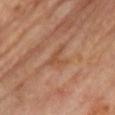{
  "patient": {
    "sex": "female",
    "age_approx": 65
  },
  "image": {
    "source": "total-body photography crop",
    "field_of_view_mm": 15
  },
  "lesion_size": {
    "long_diameter_mm_approx": 4.0
  },
  "lighting": "cross-polarized",
  "site": "chest"
}